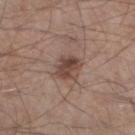Located on the left lower leg.
This image is a 15 mm lesion crop taken from a total-body photograph.
The subject is a male approximately 45 years of age.